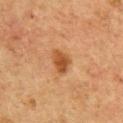The lesion was tiled from a total-body skin photograph and was not biopsied.
On the chest.
The patient is a male aged 73 to 77.
A 15 mm close-up extracted from a 3D total-body photography capture.
The recorded lesion diameter is about 3 mm.